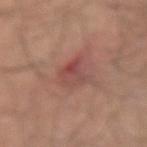{
  "biopsy_status": "not biopsied; imaged during a skin examination",
  "image": {
    "source": "total-body photography crop",
    "field_of_view_mm": 15
  },
  "site": "right upper arm",
  "patient": {
    "sex": "male",
    "age_approx": 35
  },
  "lesion_size": {
    "long_diameter_mm_approx": 3.5
  }
}